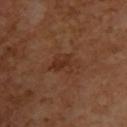Q: Was a biopsy performed?
A: catalogued during a skin exam; not biopsied
Q: Automated lesion metrics?
A: a footprint of about 7 mm²; a mean CIELAB color near L≈33 a*≈22 b*≈30, a lesion–skin lightness drop of about 6, and a normalized border contrast of about 6
Q: What is the imaging modality?
A: ~15 mm crop, total-body skin-cancer survey
Q: Lesion location?
A: the upper back
Q: What is the lesion's diameter?
A: ≈4 mm
Q: What are the patient's age and sex?
A: female, in their mid-50s
Q: What lighting was used for the tile?
A: cross-polarized illumination